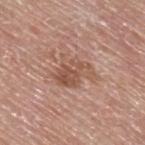Q: How was the tile lit?
A: white-light illumination
Q: Who is the patient?
A: male, approximately 80 years of age
Q: What kind of image is this?
A: 15 mm crop, total-body photography
Q: Where on the body is the lesion?
A: the upper back
Q: How large is the lesion?
A: ~5 mm (longest diameter)